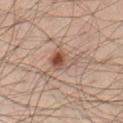* patient: male, in their mid- to late 60s
* diameter: ≈3.5 mm
* image-analysis metrics: a mean CIELAB color near L≈49 a*≈19 b*≈27; border irregularity of about 4 on a 0–10 scale, a within-lesion color-variation index near 9/10, and radial color variation of about 3; an automated nevus-likeness rating near 95 out of 100 and a lesion-detection confidence of about 100/100
* anatomic site: the abdomen
* image: total-body-photography crop, ~15 mm field of view